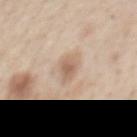Notes:
– notes: catalogued during a skin exam; not biopsied
– illumination: white-light
– body site: the abdomen
– imaging modality: ~15 mm tile from a whole-body skin photo
– patient: male, about 60 years old
– lesion diameter: ~3 mm (longest diameter)